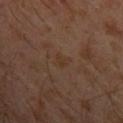Assessment:
Captured during whole-body skin photography for melanoma surveillance; the lesion was not biopsied.
Clinical summary:
The recorded lesion diameter is about 2.5 mm. A male subject, about 30 years old. From the left upper arm. Imaged with cross-polarized lighting. Cropped from a whole-body photographic skin survey; the tile spans about 15 mm.